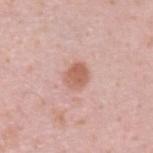No biopsy was performed on this lesion — it was imaged during a full skin examination and was not determined to be concerning.
A region of skin cropped from a whole-body photographic capture, roughly 15 mm wide.
Captured under white-light illumination.
On the upper back.
About 2.5 mm across.
A male subject, in their mid-30s.